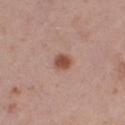Captured during whole-body skin photography for melanoma surveillance; the lesion was not biopsied. Located on the leg. A region of skin cropped from a whole-body photographic capture, roughly 15 mm wide. About 2.5 mm across. A female subject, in their 40s. Automated tile analysis of the lesion measured a lesion color around L≈51 a*≈22 b*≈28 in CIELAB, roughly 12 lightness units darker than nearby skin, and a lesion-to-skin contrast of about 9 (normalized; higher = more distinct). The analysis additionally found a border-irregularity rating of about 2/10 and peripheral color asymmetry of about 1. The analysis additionally found a classifier nevus-likeness of about 95/100 and a lesion-detection confidence of about 100/100. This is a white-light tile.